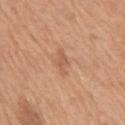Impression:
Captured during whole-body skin photography for melanoma surveillance; the lesion was not biopsied.
Background:
The lesion is located on the right upper arm. The patient is a male about 70 years old. A region of skin cropped from a whole-body photographic capture, roughly 15 mm wide.